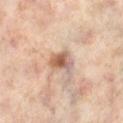Impression: The lesion was photographed on a routine skin check and not biopsied; there is no pathology result. Clinical summary: A lesion tile, about 15 mm wide, cut from a 3D total-body photograph. Automated tile analysis of the lesion measured roughly 13 lightness units darker than nearby skin and a normalized border contrast of about 8.5. The analysis additionally found an automated nevus-likeness rating near 85 out of 100. A female patient aged approximately 50. This is a cross-polarized tile. Approximately 3 mm at its widest. Located on the leg.